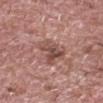workup: total-body-photography surveillance lesion; no biopsy | subject: male, aged 68–72 | tile lighting: white-light | anatomic site: the head or neck | acquisition: ~15 mm crop, total-body skin-cancer survey.